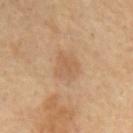Captured during whole-body skin photography for melanoma surveillance; the lesion was not biopsied.
A region of skin cropped from a whole-body photographic capture, roughly 15 mm wide.
Captured under cross-polarized illumination.
About 3.5 mm across.
From the mid back.
Automated image analysis of the tile measured a footprint of about 8 mm², an outline eccentricity of about 0.65 (0 = round, 1 = elongated), and a symmetry-axis asymmetry near 0.2. It also reported a mean CIELAB color near L≈57 a*≈18 b*≈33, about 7 CIELAB-L* units darker than the surrounding skin, and a normalized lesion–skin contrast near 4.5. The software also gave a lesion-detection confidence of about 100/100.
A male subject, approximately 70 years of age.